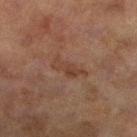| field | value |
|---|---|
| biopsy status | imaged on a skin check; not biopsied |
| body site | the left lower leg |
| automated metrics | border irregularity of about 5 on a 0–10 scale, internal color variation of about 2.5 on a 0–10 scale, and a peripheral color-asymmetry measure near 1; a classifier nevus-likeness of about 0/100 and a lesion-detection confidence of about 100/100 |
| subject | female, aged around 60 |
| image source | total-body-photography crop, ~15 mm field of view |
| tile lighting | cross-polarized illumination |
| diameter | ≈4 mm |